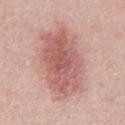| feature | finding |
|---|---|
| workup | catalogued during a skin exam; not biopsied |
| image-analysis metrics | a lesion area of about 36 mm² and a shape eccentricity near 0.85; an average lesion color of about L≈59 a*≈24 b*≈24 (CIELAB), about 12 CIELAB-L* units darker than the surrounding skin, and a lesion-to-skin contrast of about 7.5 (normalized; higher = more distinct) |
| body site | the chest |
| acquisition | 15 mm crop, total-body photography |
| subject | male, approximately 55 years of age |
| illumination | white-light illumination |